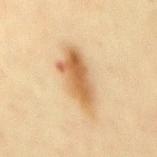Recorded during total-body skin imaging; not selected for excision or biopsy. The recorded lesion diameter is about 6.5 mm. An algorithmic analysis of the crop reported roughly 12 lightness units darker than nearby skin and a lesion-to-skin contrast of about 8.5 (normalized; higher = more distinct). And it measured a classifier nevus-likeness of about 90/100 and a lesion-detection confidence of about 100/100. A female patient, in their mid-40s. The lesion is on the mid back. A lesion tile, about 15 mm wide, cut from a 3D total-body photograph.